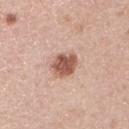biopsy_status: not biopsied; imaged during a skin examination
patient:
  sex: male
  age_approx: 45
site: left upper arm
image:
  source: total-body photography crop
  field_of_view_mm: 15